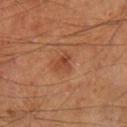biopsy status: no biopsy performed (imaged during a skin exam)
image-analysis metrics: an average lesion color of about L≈39 a*≈23 b*≈31 (CIELAB), roughly 7 lightness units darker than nearby skin, and a normalized lesion–skin contrast near 6; a border-irregularity rating of about 4/10, a color-variation rating of about 2/10, and peripheral color asymmetry of about 0.5
image source: total-body-photography crop, ~15 mm field of view
location: the left lower leg
lighting: cross-polarized illumination
subject: male, in their mid- to late 60s